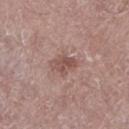Recorded during total-body skin imaging; not selected for excision or biopsy.
Cropped from a whole-body photographic skin survey; the tile spans about 15 mm.
The tile uses white-light illumination.
About 3.5 mm across.
A female subject, in their mid- to late 60s.
The lesion is on the leg.